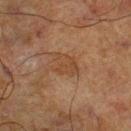Case summary:
- workup — catalogued during a skin exam; not biopsied
- image-analysis metrics — a classifier nevus-likeness of about 0/100
- patient — male, aged approximately 70
- tile lighting — cross-polarized
- acquisition — 15 mm crop, total-body photography
- lesion size — ~3.5 mm (longest diameter)
- location — the right lower leg